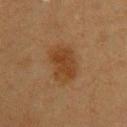This lesion was catalogued during total-body skin photography and was not selected for biopsy. Imaged with cross-polarized lighting. Cropped from a whole-body photographic skin survey; the tile spans about 15 mm. The recorded lesion diameter is about 5 mm. The lesion is on the upper back. The patient is a female roughly 40 years of age. Automated tile analysis of the lesion measured a border-irregularity index near 2/10, internal color variation of about 3 on a 0–10 scale, and radial color variation of about 1. The software also gave a detector confidence of about 100 out of 100 that the crop contains a lesion.